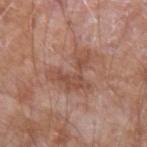{
  "lesion_size": {
    "long_diameter_mm_approx": 5.0
  },
  "automated_metrics": {
    "border_irregularity_0_10": 9.0,
    "color_variation_0_10": 3.0,
    "lesion_detection_confidence_0_100": 100
  },
  "image": {
    "source": "total-body photography crop",
    "field_of_view_mm": 15
  },
  "patient": {
    "sex": "male",
    "age_approx": 60
  },
  "site": "left forearm",
  "lighting": "white-light"
}